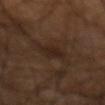| field | value |
|---|---|
| follow-up | no biopsy performed (imaged during a skin exam) |
| automated lesion analysis | border irregularity of about 2 on a 0–10 scale, internal color variation of about 2.5 on a 0–10 scale, and a peripheral color-asymmetry measure near 1 |
| body site | the arm |
| patient | male, approximately 60 years of age |
| acquisition | 15 mm crop, total-body photography |
| illumination | cross-polarized illumination |